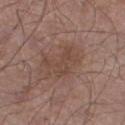<case>
  <image>
    <source>total-body photography crop</source>
    <field_of_view_mm>15</field_of_view_mm>
  </image>
  <site>leg</site>
  <patient>
    <sex>male</sex>
    <age_approx>55</age_approx>
  </patient>
  <automated_metrics>
    <area_mm2_approx>8.5</area_mm2_approx>
    <shape_asymmetry>0.55</shape_asymmetry>
    <vs_skin_darker_L>6.0</vs_skin_darker_L>
    <vs_skin_contrast_norm>5.0</vs_skin_contrast_norm>
    <color_variation_0_10>2.0</color_variation_0_10>
    <peripheral_color_asymmetry>1.0</peripheral_color_asymmetry>
  </automated_metrics>
</case>